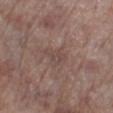Impression:
Imaged during a routine full-body skin examination; the lesion was not biopsied and no histopathology is available.
Acquisition and patient details:
Cropped from a total-body skin-imaging series; the visible field is about 15 mm. From the leg. This is a white-light tile. About 3 mm across. Automated tile analysis of the lesion measured a footprint of about 4 mm² and a shape eccentricity near 0.8. The software also gave a border-irregularity rating of about 4/10, internal color variation of about 2 on a 0–10 scale, and peripheral color asymmetry of about 0.5. The analysis additionally found an automated nevus-likeness rating near 0 out of 100 and lesion-presence confidence of about 95/100. A male patient, aged around 70.